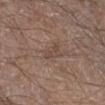Assessment: The lesion was tiled from a total-body skin photograph and was not biopsied. Acquisition and patient details: Approximately 3 mm at its widest. Captured under white-light illumination. An algorithmic analysis of the crop reported a footprint of about 3 mm². The analysis additionally found a border-irregularity rating of about 4.5/10 and radial color variation of about 0. And it measured an automated nevus-likeness rating near 0 out of 100 and lesion-presence confidence of about 95/100. From the right lower leg. A male subject aged around 60. A 15 mm crop from a total-body photograph taken for skin-cancer surveillance.